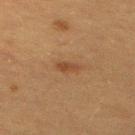Findings:
- workup · total-body-photography surveillance lesion; no biopsy
- image-analysis metrics · a lesion-to-skin contrast of about 6.5 (normalized; higher = more distinct); a border-irregularity rating of about 2.5/10, internal color variation of about 1.5 on a 0–10 scale, and radial color variation of about 0.5
- tile lighting · cross-polarized
- size · about 2.5 mm
- body site · the upper back
- acquisition · ~15 mm tile from a whole-body skin photo
- patient · female, roughly 55 years of age